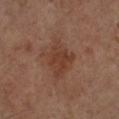Notes:
• workup — no biopsy performed (imaged during a skin exam)
• location — the left lower leg
• tile lighting — cross-polarized
• subject — male, about 65 years old
• lesion diameter — ≈4 mm
• image source — ~15 mm tile from a whole-body skin photo